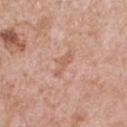Captured during whole-body skin photography for melanoma surveillance; the lesion was not biopsied. On the chest. Automated image analysis of the tile measured an area of roughly 4 mm², an outline eccentricity of about 0.95 (0 = round, 1 = elongated), and two-axis asymmetry of about 0.4. And it measured an automated nevus-likeness rating near 0 out of 100 and lesion-presence confidence of about 100/100. A close-up tile cropped from a whole-body skin photograph, about 15 mm across. The subject is a male aged 58 to 62.